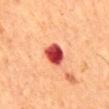{"lighting": "cross-polarized", "site": "mid back", "lesion_size": {"long_diameter_mm_approx": 3.0}, "image": {"source": "total-body photography crop", "field_of_view_mm": 15}, "patient": {"sex": "male", "age_approx": 65}}